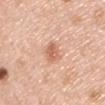Recorded during total-body skin imaging; not selected for excision or biopsy. The patient is a male approximately 40 years of age. The tile uses white-light illumination. An algorithmic analysis of the crop reported a lesion area of about 5 mm² and a symmetry-axis asymmetry near 0.25. It also reported a lesion–skin lightness drop of about 11 and a normalized border contrast of about 6.5. The analysis additionally found a border-irregularity rating of about 2.5/10 and a color-variation rating of about 3/10. A region of skin cropped from a whole-body photographic capture, roughly 15 mm wide. Located on the left upper arm. The recorded lesion diameter is about 3 mm.